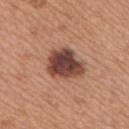follow-up: total-body-photography surveillance lesion; no biopsy | TBP lesion metrics: a footprint of about 14 mm² and a shape-asymmetry score of about 0.2 (0 = symmetric); an average lesion color of about L≈44 a*≈22 b*≈25 (CIELAB) and a lesion-to-skin contrast of about 12.5 (normalized; higher = more distinct); a nevus-likeness score of about 15/100 | tile lighting: white-light | lesion diameter: ~4.5 mm (longest diameter) | patient: female, aged 33–37 | image source: total-body-photography crop, ~15 mm field of view | location: the right upper arm.